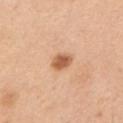{"biopsy_status": "not biopsied; imaged during a skin examination", "site": "chest", "patient": {"sex": "male", "age_approx": 50}, "image": {"source": "total-body photography crop", "field_of_view_mm": 15}}